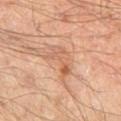Case summary:
– anatomic site: the left thigh
– automated metrics: a mean CIELAB color near L≈60 a*≈23 b*≈34, roughly 8 lightness units darker than nearby skin, and a normalized border contrast of about 5.5
– lesion diameter: ≈4.5 mm
– acquisition: ~15 mm crop, total-body skin-cancer survey
– patient: male, in their mid- to late 40s
– lighting: cross-polarized illumination